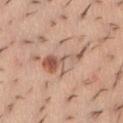Clinical impression:
Imaged during a routine full-body skin examination; the lesion was not biopsied and no histopathology is available.
Clinical summary:
The total-body-photography lesion software estimated a lesion area of about 9.5 mm² and an outline eccentricity of about 0.9 (0 = round, 1 = elongated). And it measured a mean CIELAB color near L≈58 a*≈20 b*≈29 and a normalized lesion–skin contrast near 7. And it measured a border-irregularity index near 8/10 and a color-variation rating of about 7.5/10. It also reported a detector confidence of about 100 out of 100 that the crop contains a lesion. Imaged with white-light lighting. Cropped from a whole-body photographic skin survey; the tile spans about 15 mm. A female patient in their mid-20s. The lesion is on the abdomen.